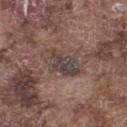Part of a total-body skin-imaging series; this lesion was reviewed on a skin check and was not flagged for biopsy. A 15 mm crop from a total-body photograph taken for skin-cancer surveillance. A male patient in their mid-70s. From the left thigh.